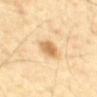notes: imaged on a skin check; not biopsied.